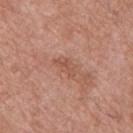workup: imaged on a skin check; not biopsied | imaging modality: total-body-photography crop, ~15 mm field of view | automated metrics: a border-irregularity index near 5/10, internal color variation of about 1.5 on a 0–10 scale, and radial color variation of about 0.5; an automated nevus-likeness rating near 0 out of 100 and lesion-presence confidence of about 100/100 | subject: male, in their mid-60s | tile lighting: white-light | body site: the upper back | size: ~3 mm (longest diameter).